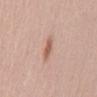subject: female, in their mid- to late 50s; image source: total-body-photography crop, ~15 mm field of view; image-analysis metrics: an outline eccentricity of about 0.8 (0 = round, 1 = elongated); lesion size: ~2.5 mm (longest diameter); location: the mid back; lighting: white-light.